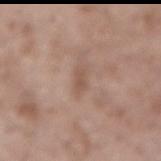biopsy status: imaged on a skin check; not biopsied | image-analysis metrics: a footprint of about 2.5 mm² and an outline eccentricity of about 0.9 (0 = round, 1 = elongated); a lesion-to-skin contrast of about 5.5 (normalized; higher = more distinct) | diameter: ≈2.5 mm | acquisition: ~15 mm crop, total-body skin-cancer survey | subject: male, aged around 55 | site: the lower back.